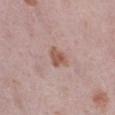Q: Was a biopsy performed?
A: total-body-photography surveillance lesion; no biopsy
Q: Patient demographics?
A: female, aged 38 to 42
Q: What is the anatomic site?
A: the left lower leg
Q: What kind of image is this?
A: ~15 mm tile from a whole-body skin photo